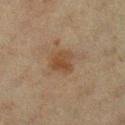tile lighting: cross-polarized illumination; lesion size: ≈3 mm; subject: female, aged approximately 40; site: the left lower leg; acquisition: total-body-photography crop, ~15 mm field of view.